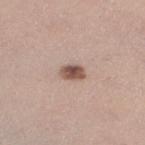Assessment: The lesion was tiled from a total-body skin photograph and was not biopsied. Clinical summary: This image is a 15 mm lesion crop taken from a total-body photograph. A female patient roughly 40 years of age. The lesion's longest dimension is about 2.5 mm. This is a white-light tile. From the left lower leg.